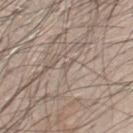notes: no biopsy performed (imaged during a skin exam); lesion size: ≈1 mm; imaging modality: 15 mm crop, total-body photography; patient: male, approximately 45 years of age; tile lighting: white-light; body site: the chest.